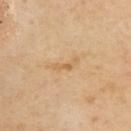This lesion was catalogued during total-body skin photography and was not selected for biopsy. A 15 mm close-up tile from a total-body photography series done for melanoma screening. Captured under cross-polarized illumination. The lesion's longest dimension is about 3.5 mm. The total-body-photography lesion software estimated an area of roughly 3 mm², an eccentricity of roughly 0.95, and a symmetry-axis asymmetry near 0.6. It also reported a nevus-likeness score of about 0/100 and a lesion-detection confidence of about 100/100. The subject is a male approximately 55 years of age. The lesion is on the back.